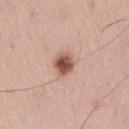The lesion was photographed on a routine skin check and not biopsied; there is no pathology result.
An algorithmic analysis of the crop reported an average lesion color of about L≈54 a*≈22 b*≈28 (CIELAB), about 16 CIELAB-L* units darker than the surrounding skin, and a normalized border contrast of about 10.5. The analysis additionally found an automated nevus-likeness rating near 100 out of 100.
The lesion is on the lower back.
The recorded lesion diameter is about 2.5 mm.
A male subject, aged approximately 55.
Captured under white-light illumination.
A region of skin cropped from a whole-body photographic capture, roughly 15 mm wide.